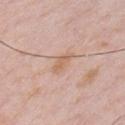notes: no biopsy performed (imaged during a skin exam)
anatomic site: the chest
lesion diameter: ~3 mm (longest diameter)
subject: male, in their mid- to late 30s
acquisition: 15 mm crop, total-body photography